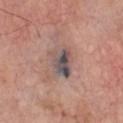The lesion was tiled from a total-body skin photograph and was not biopsied. A male patient about 60 years old. Cropped from a total-body skin-imaging series; the visible field is about 15 mm. On the chest.